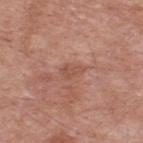notes: no biopsy performed (imaged during a skin exam)
diameter: about 2.5 mm
acquisition: 15 mm crop, total-body photography
patient: male, in their mid-50s
tile lighting: white-light illumination
image-analysis metrics: an outline eccentricity of about 0.85 (0 = round, 1 = elongated); a detector confidence of about 100 out of 100 that the crop contains a lesion
anatomic site: the upper back